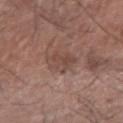biopsy_status: not biopsied; imaged during a skin examination
automated_metrics:
  area_mm2_approx: 8.5
  eccentricity: 0.65
  shape_asymmetry: 0.2
  cielab_L: 46
  cielab_a: 18
  cielab_b: 23
  vs_skin_contrast_norm: 5.5
  nevus_likeness_0_100: 0
  lesion_detection_confidence_0_100: 100
site: arm
lesion_size:
  long_diameter_mm_approx: 3.5
lighting: white-light
patient:
  sex: male
  age_approx: 65
image:
  source: total-body photography crop
  field_of_view_mm: 15